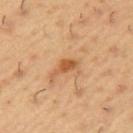{"biopsy_status": "not biopsied; imaged during a skin examination", "lighting": "cross-polarized", "patient": {"sex": "male", "age_approx": 55}, "automated_metrics": {"nevus_likeness_0_100": 70, "lesion_detection_confidence_0_100": 100}, "image": {"source": "total-body photography crop", "field_of_view_mm": 15}, "site": "left upper arm"}